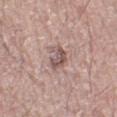Automated image analysis of the tile measured a lesion area of about 5 mm², an eccentricity of roughly 0.5, and a shape-asymmetry score of about 0.4 (0 = symmetric). And it measured an average lesion color of about L≈53 a*≈18 b*≈21 (CIELAB). And it measured an automated nevus-likeness rating near 10 out of 100 and lesion-presence confidence of about 100/100. A male subject in their 70s. Approximately 2.5 mm at its widest. The tile uses white-light illumination. A close-up tile cropped from a whole-body skin photograph, about 15 mm across. From the right leg.